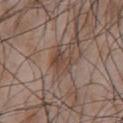Q: Was this lesion biopsied?
A: total-body-photography surveillance lesion; no biopsy
Q: How was this image acquired?
A: ~15 mm tile from a whole-body skin photo
Q: Patient demographics?
A: male, about 50 years old
Q: Where on the body is the lesion?
A: the chest
Q: What did automated image analysis measure?
A: an eccentricity of roughly 0.7 and a shape-asymmetry score of about 0.35 (0 = symmetric); a nevus-likeness score of about 15/100 and a detector confidence of about 100 out of 100 that the crop contains a lesion